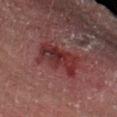{"biopsy_status": "not biopsied; imaged during a skin examination", "patient": {"sex": "male", "age_approx": 55}, "image": {"source": "total-body photography crop", "field_of_view_mm": 15}, "site": "left upper arm"}